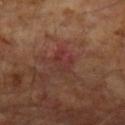The lesion was tiled from a total-body skin photograph and was not biopsied.
The lesion's longest dimension is about 3 mm.
Captured under cross-polarized illumination.
The lesion is on the left upper arm.
The patient is a male about 65 years old.
A 15 mm close-up tile from a total-body photography series done for melanoma screening.